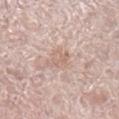A female patient aged 63 to 67.
The lesion is located on the left leg.
Imaged with white-light lighting.
Cropped from a total-body skin-imaging series; the visible field is about 15 mm.
Approximately 3 mm at its widest.